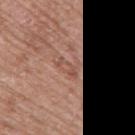Case summary:
• follow-up — total-body-photography surveillance lesion; no biopsy
• subject — female, about 60 years old
• image source — ~15 mm tile from a whole-body skin photo
• body site — the right upper arm
• lesion size — ~3 mm (longest diameter)
• automated lesion analysis — a shape eccentricity near 0.9 and a shape-asymmetry score of about 0.3 (0 = symmetric); a border-irregularity index near 4/10, a color-variation rating of about 1/10, and radial color variation of about 0
• illumination — white-light illumination